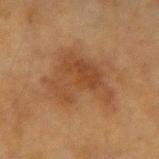biopsy status=imaged on a skin check; not biopsied | illumination=cross-polarized illumination | diameter=about 7.5 mm | body site=the arm | imaging modality=15 mm crop, total-body photography | subject=female, in their mid- to late 50s.